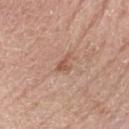Assessment: No biopsy was performed on this lesion — it was imaged during a full skin examination and was not determined to be concerning. Context: About 3 mm across. The tile uses white-light illumination. A roughly 15 mm field-of-view crop from a total-body skin photograph. A female subject, about 60 years old. On the left upper arm.